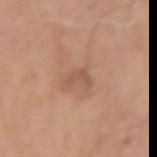workup: total-body-photography surveillance lesion; no biopsy
imaging modality: ~15 mm crop, total-body skin-cancer survey
subject: male, aged around 80
site: the right upper arm